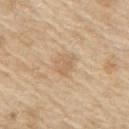{
  "image": {
    "source": "total-body photography crop",
    "field_of_view_mm": 15
  },
  "patient": {
    "sex": "male",
    "age_approx": 70
  },
  "site": "right upper arm"
}